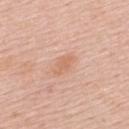Impression: Recorded during total-body skin imaging; not selected for excision or biopsy. Clinical summary: A 15 mm close-up tile from a total-body photography series done for melanoma screening. The patient is a male aged 53–57. The total-body-photography lesion software estimated a footprint of about 3.5 mm² and two-axis asymmetry of about 0.3. It also reported an average lesion color of about L≈65 a*≈22 b*≈33 (CIELAB) and a normalized lesion–skin contrast near 5.5. And it measured border irregularity of about 3 on a 0–10 scale and a color-variation rating of about 1.5/10. And it measured lesion-presence confidence of about 100/100. From the upper back. Longest diameter approximately 2.5 mm.